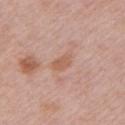Impression: The lesion was photographed on a routine skin check and not biopsied; there is no pathology result. Image and clinical context: A female patient in their 60s. This is a white-light tile. The lesion is on the left upper arm. A 15 mm crop from a total-body photograph taken for skin-cancer surveillance.